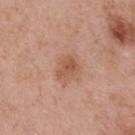workup — imaged on a skin check; not biopsied
TBP lesion metrics — an area of roughly 4.5 mm² and a shape-asymmetry score of about 0.35 (0 = symmetric); a lesion color around L≈54 a*≈23 b*≈32 in CIELAB, a lesion–skin lightness drop of about 9, and a normalized border contrast of about 6.5; a classifier nevus-likeness of about 35/100
patient — male, aged around 55
image source — 15 mm crop, total-body photography
anatomic site — the back